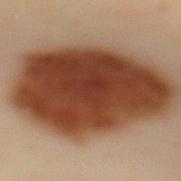{
  "site": "mid back",
  "image": {
    "source": "total-body photography crop",
    "field_of_view_mm": 15
  },
  "lesion_size": {
    "long_diameter_mm_approx": 12.0
  },
  "patient": {
    "sex": "female",
    "age_approx": 60
  }
}